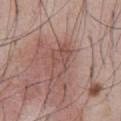biopsy_status: not biopsied; imaged during a skin examination
site: abdomen
image:
  source: total-body photography crop
  field_of_view_mm: 15
lighting: white-light
lesion_size:
  long_diameter_mm_approx: 4.5
patient:
  sex: male
  age_approx: 55